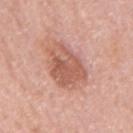The lesion was photographed on a routine skin check and not biopsied; there is no pathology result. On the back. A male subject in their 60s. Longest diameter approximately 5 mm. A roughly 15 mm field-of-view crop from a total-body skin photograph. This is a white-light tile.